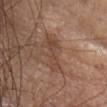{"biopsy_status": "not biopsied; imaged during a skin examination", "lighting": "white-light", "site": "head or neck", "lesion_size": {"long_diameter_mm_approx": 5.0}, "automated_metrics": {"area_mm2_approx": 6.5, "eccentricity": 0.95, "shape_asymmetry": 0.5, "cielab_L": 45, "cielab_a": 19, "cielab_b": 28, "vs_skin_darker_L": 7.0, "vs_skin_contrast_norm": 6.0, "border_irregularity_0_10": 6.5, "peripheral_color_asymmetry": 1.0, "nevus_likeness_0_100": 0}, "image": {"source": "total-body photography crop", "field_of_view_mm": 15}, "patient": {"sex": "female", "age_approx": 75}}